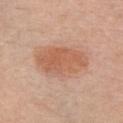Clinical impression:
No biopsy was performed on this lesion — it was imaged during a full skin examination and was not determined to be concerning.
Context:
Captured under white-light illumination. The lesion is located on the front of the torso. A 15 mm close-up extracted from a 3D total-body photography capture. A female subject, approximately 60 years of age.